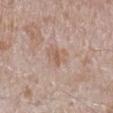Recorded during total-body skin imaging; not selected for excision or biopsy.
The subject is a male aged 43 to 47.
The total-body-photography lesion software estimated border irregularity of about 3 on a 0–10 scale, internal color variation of about 3 on a 0–10 scale, and radial color variation of about 1. It also reported a nevus-likeness score of about 0/100.
On the right lower leg.
A region of skin cropped from a whole-body photographic capture, roughly 15 mm wide.
This is a white-light tile.